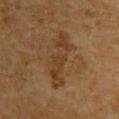Findings:
– biopsy status — imaged on a skin check; not biopsied
– patient — male, roughly 85 years of age or older
– lesion size — about 7 mm
– location — the upper back
– automated metrics — a lesion–skin lightness drop of about 6 and a lesion-to-skin contrast of about 6.5 (normalized; higher = more distinct); internal color variation of about 2.5 on a 0–10 scale and peripheral color asymmetry of about 1; a nevus-likeness score of about 0/100 and a lesion-detection confidence of about 100/100
– image — 15 mm crop, total-body photography